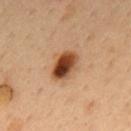Case summary:
– workup — catalogued during a skin exam; not biopsied
– location — the back
– patient — male, in their 50s
– tile lighting — cross-polarized illumination
– image source — 15 mm crop, total-body photography
– diameter — about 3.5 mm
– automated metrics — a lesion–skin lightness drop of about 18 and a lesion-to-skin contrast of about 13 (normalized; higher = more distinct); a border-irregularity rating of about 2/10, a within-lesion color-variation index near 10/10, and radial color variation of about 4